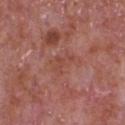image:
  source: total-body photography crop
  field_of_view_mm: 15
lesion_size:
  long_diameter_mm_approx: 3.0
lighting: white-light
patient:
  sex: male
  age_approx: 65
site: front of the torso